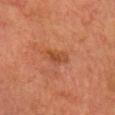workup — imaged on a skin check; not biopsied